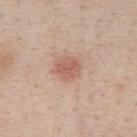Findings:
• biopsy status · catalogued during a skin exam; not biopsied
• site · the front of the torso
• lesion size · ≈3 mm
• imaging modality · ~15 mm tile from a whole-body skin photo
• lighting · white-light
• subject · male, aged 58 to 62